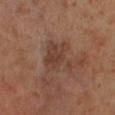Findings:
– follow-up — total-body-photography surveillance lesion; no biopsy
– subject — male, in their 70s
– body site — the right lower leg
– automated metrics — a footprint of about 11 mm², a shape eccentricity near 0.45, and a symmetry-axis asymmetry near 0.5; a classifier nevus-likeness of about 0/100 and a lesion-detection confidence of about 100/100
– image — 15 mm crop, total-body photography